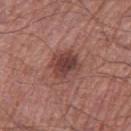<record>
  <biopsy_status>not biopsied; imaged during a skin examination</biopsy_status>
  <patient>
    <sex>male</sex>
    <age_approx>70</age_approx>
  </patient>
  <site>right thigh</site>
  <image>
    <source>total-body photography crop</source>
    <field_of_view_mm>15</field_of_view_mm>
  </image>
</record>